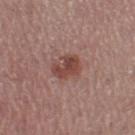| field | value |
|---|---|
| workup | imaged on a skin check; not biopsied |
| automated metrics | a lesion area of about 6.5 mm²; border irregularity of about 3 on a 0–10 scale |
| acquisition | ~15 mm tile from a whole-body skin photo |
| size | ≈3.5 mm |
| tile lighting | white-light illumination |
| body site | the left thigh |
| subject | female, aged around 55 |